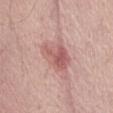Findings:
– workup: total-body-photography surveillance lesion; no biopsy
– body site: the abdomen
– diameter: about 4 mm
– automated lesion analysis: a border-irregularity rating of about 3.5/10, internal color variation of about 5 on a 0–10 scale, and peripheral color asymmetry of about 1.5
– lighting: white-light
– image: ~15 mm crop, total-body skin-cancer survey
– patient: male, in their mid- to late 60s